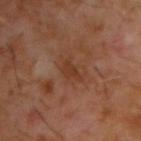notes: total-body-photography surveillance lesion; no biopsy
acquisition: ~15 mm tile from a whole-body skin photo
subject: male, aged approximately 60
location: the upper back
lighting: cross-polarized illumination